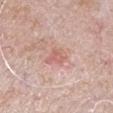Q: Was this lesion biopsied?
A: no biopsy performed (imaged during a skin exam)
Q: Automated lesion metrics?
A: a footprint of about 5.5 mm², a shape eccentricity near 0.5, and a shape-asymmetry score of about 0.3 (0 = symmetric); border irregularity of about 3 on a 0–10 scale and a within-lesion color-variation index near 4/10
Q: How was the tile lit?
A: white-light
Q: Patient demographics?
A: male, aged 63 to 67
Q: Lesion location?
A: the arm
Q: How was this image acquired?
A: 15 mm crop, total-body photography